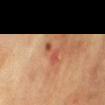The lesion was photographed on a routine skin check and not biopsied; there is no pathology result. A roughly 15 mm field-of-view crop from a total-body skin photograph. This is a cross-polarized tile. Approximately 3 mm at its widest. The lesion is on the chest. The subject is a female aged around 50.